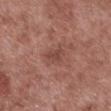<lesion>
  <biopsy_status>not biopsied; imaged during a skin examination</biopsy_status>
  <lighting>white-light</lighting>
  <lesion_size>
    <long_diameter_mm_approx>2.5</long_diameter_mm_approx>
  </lesion_size>
  <patient>
    <sex>male</sex>
    <age_approx>55</age_approx>
  </patient>
  <site>leg</site>
  <image>
    <source>total-body photography crop</source>
    <field_of_view_mm>15</field_of_view_mm>
  </image>
  <automated_metrics>
    <cielab_L>45</cielab_L>
    <cielab_a>23</cielab_a>
    <cielab_b>24</cielab_b>
    <vs_skin_darker_L>8.0</vs_skin_darker_L>
    <vs_skin_contrast_norm>6.0</vs_skin_contrast_norm>
    <border_irregularity_0_10>3.0</border_irregularity_0_10>
    <color_variation_0_10>1.0</color_variation_0_10>
    <peripheral_color_asymmetry>0.5</peripheral_color_asymmetry>
  </automated_metrics>
</lesion>